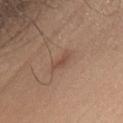The lesion is located on the front of the torso.
A 15 mm close-up extracted from a 3D total-body photography capture.
The tile uses white-light illumination.
The lesion's longest dimension is about 3 mm.
A male patient, aged around 30.
An algorithmic analysis of the crop reported a symmetry-axis asymmetry near 0.4. And it measured a lesion color around L≈48 a*≈20 b*≈27 in CIELAB, roughly 7 lightness units darker than nearby skin, and a normalized lesion–skin contrast near 5.5.